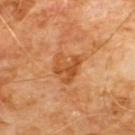The lesion was photographed on a routine skin check and not biopsied; there is no pathology result.
The lesion is on the chest.
The patient is a male roughly 60 years of age.
A region of skin cropped from a whole-body photographic capture, roughly 15 mm wide.
Imaged with cross-polarized lighting.